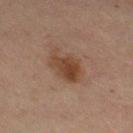follow-up: no biopsy performed (imaged during a skin exam) | lighting: cross-polarized illumination | lesion diameter: ~4.5 mm (longest diameter) | site: the right thigh | image source: ~15 mm tile from a whole-body skin photo | patient: female, approximately 70 years of age.